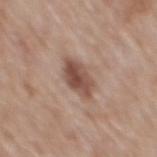Q: Was this lesion biopsied?
A: imaged on a skin check; not biopsied
Q: What lighting was used for the tile?
A: white-light illumination
Q: What did automated image analysis measure?
A: a lesion area of about 8 mm², an outline eccentricity of about 0.8 (0 = round, 1 = elongated), and a symmetry-axis asymmetry near 0.2; a border-irregularity rating of about 2/10 and radial color variation of about 1.5; a classifier nevus-likeness of about 70/100 and lesion-presence confidence of about 100/100
Q: Where on the body is the lesion?
A: the mid back
Q: Lesion size?
A: about 4.5 mm
Q: What are the patient's age and sex?
A: male, aged 58 to 62
Q: What is the imaging modality?
A: ~15 mm crop, total-body skin-cancer survey